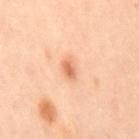body site — the left leg
imaging modality — ~15 mm crop, total-body skin-cancer survey
patient — female, aged approximately 40